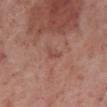Assessment: This lesion was catalogued during total-body skin photography and was not selected for biopsy. Context: This image is a 15 mm lesion crop taken from a total-body photograph. The lesion is located on the right lower leg. A male patient aged 68 to 72.